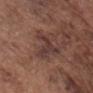  biopsy_status: not biopsied; imaged during a skin examination
  site: head or neck
  automated_metrics:
    cielab_L: 36
    cielab_a: 18
    cielab_b: 20
    vs_skin_darker_L: 8.0
    border_irregularity_0_10: 8.5
    color_variation_0_10: 2.0
    peripheral_color_asymmetry: 0.5
  image:
    source: total-body photography crop
    field_of_view_mm: 15
  lighting: white-light
  patient:
    sex: male
    age_approx: 75